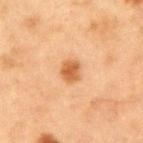Q: Is there a histopathology result?
A: total-body-photography surveillance lesion; no biopsy
Q: Illumination type?
A: cross-polarized
Q: What did automated image analysis measure?
A: a footprint of about 4.5 mm², a shape eccentricity near 0.7, and a symmetry-axis asymmetry near 0.2; an average lesion color of about L≈52 a*≈23 b*≈38 (CIELAB), roughly 12 lightness units darker than nearby skin, and a normalized border contrast of about 8.5
Q: What is the anatomic site?
A: the arm
Q: Who is the patient?
A: female, aged 38–42
Q: What kind of image is this?
A: ~15 mm tile from a whole-body skin photo